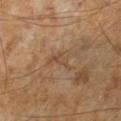Clinical impression: The lesion was tiled from a total-body skin photograph and was not biopsied. Image and clinical context: This image is a 15 mm lesion crop taken from a total-body photograph. A male patient roughly 65 years of age. The tile uses cross-polarized illumination. Approximately 3 mm at its widest.